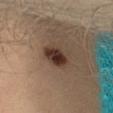Image and clinical context:
A 15 mm crop from a total-body photograph taken for skin-cancer surveillance. A male patient, aged 53–57. The tile uses cross-polarized illumination. On the left thigh. Measured at roughly 4 mm in maximum diameter. An algorithmic analysis of the crop reported a symmetry-axis asymmetry near 0.2. The analysis additionally found a mean CIELAB color near L≈33 a*≈16 b*≈23 and about 13 CIELAB-L* units darker than the surrounding skin. The analysis additionally found radial color variation of about 2. The analysis additionally found a classifier nevus-likeness of about 100/100 and a lesion-detection confidence of about 100/100.